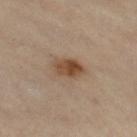biopsy_status: not biopsied; imaged during a skin examination
lesion_size:
  long_diameter_mm_approx: 3.5
automated_metrics:
  cielab_L: 46
  cielab_a: 18
  cielab_b: 30
  vs_skin_darker_L: 11.0
  vs_skin_contrast_norm: 9.5
  border_irregularity_0_10: 2.0
  color_variation_0_10: 5.5
  peripheral_color_asymmetry: 2.0
  nevus_likeness_0_100: 95
  lesion_detection_confidence_0_100: 100
site: leg
image:
  source: total-body photography crop
  field_of_view_mm: 15
lighting: cross-polarized
patient:
  sex: female
  age_approx: 60